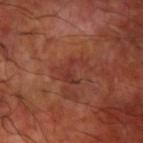Recorded during total-body skin imaging; not selected for excision or biopsy.
Imaged with cross-polarized lighting.
A male patient in their 60s.
Approximately 3.5 mm at its widest.
A lesion tile, about 15 mm wide, cut from a 3D total-body photograph.
Automated image analysis of the tile measured a lesion color around L≈35 a*≈27 b*≈27 in CIELAB and about 6 CIELAB-L* units darker than the surrounding skin.
The lesion is on the arm.